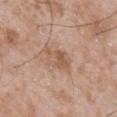biopsy status: no biopsy performed (imaged during a skin exam)
lesion size: about 4 mm
body site: the mid back
acquisition: total-body-photography crop, ~15 mm field of view
lighting: white-light
subject: male, in their 50s